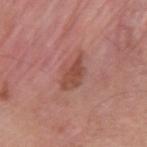Notes:
* workup — catalogued during a skin exam; not biopsied
* lesion diameter — about 4.5 mm
* subject — male, roughly 40 years of age
* lighting — white-light illumination
* imaging modality — 15 mm crop, total-body photography
* site — the upper back